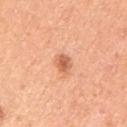notes: total-body-photography surveillance lesion; no biopsy | automated lesion analysis: a mean CIELAB color near L≈63 a*≈28 b*≈37, about 12 CIELAB-L* units darker than the surrounding skin, and a normalized lesion–skin contrast near 7.5 | site: the left upper arm | patient: male, in their 50s | imaging modality: ~15 mm tile from a whole-body skin photo | tile lighting: white-light | diameter: ≈2.5 mm.